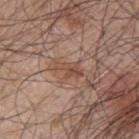{
  "biopsy_status": "not biopsied; imaged during a skin examination",
  "image": {
    "source": "total-body photography crop",
    "field_of_view_mm": 15
  },
  "patient": {
    "sex": "male",
    "age_approx": 50
  },
  "site": "upper back",
  "lesion_size": {
    "long_diameter_mm_approx": 3.5
  }
}